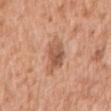Clinical impression:
No biopsy was performed on this lesion — it was imaged during a full skin examination and was not determined to be concerning.
Background:
The lesion is located on the right upper arm. A 15 mm close-up extracted from a 3D total-body photography capture. A female patient approximately 40 years of age.